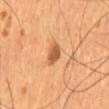Impression:
No biopsy was performed on this lesion — it was imaged during a full skin examination and was not determined to be concerning.
Clinical summary:
Cropped from a total-body skin-imaging series; the visible field is about 15 mm. A male patient aged 53 to 57. The lesion is located on the abdomen.